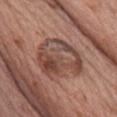notes — total-body-photography surveillance lesion; no biopsy | image — total-body-photography crop, ~15 mm field of view | site — the chest | diameter — about 6.5 mm | TBP lesion metrics — a footprint of about 23 mm², an outline eccentricity of about 0.6 (0 = round, 1 = elongated), and two-axis asymmetry of about 0.25; a border-irregularity rating of about 3/10, internal color variation of about 9 on a 0–10 scale, and a peripheral color-asymmetry measure near 3 | lighting — white-light illumination | patient — female, approximately 60 years of age.